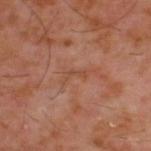The lesion was photographed on a routine skin check and not biopsied; there is no pathology result. A male subject, about 60 years old. Cropped from a whole-body photographic skin survey; the tile spans about 15 mm. From the upper back.